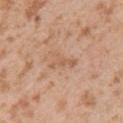* image-analysis metrics · a normalized lesion–skin contrast near 5; a nevus-likeness score of about 0/100 and a lesion-detection confidence of about 100/100
* imaging modality · ~15 mm crop, total-body skin-cancer survey
* site · the left upper arm
* lighting · white-light
* subject · male, aged around 25